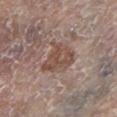Recorded during total-body skin imaging; not selected for excision or biopsy. A female patient aged approximately 85 or older. Longest diameter approximately 4 mm. This image is a 15 mm lesion crop taken from a total-body photograph. The lesion is located on the left lower leg.